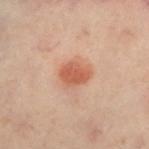Clinical summary:
About 3.5 mm across. From the right leg. A female subject, about 60 years old. Captured under cross-polarized illumination. Automated tile analysis of the lesion measured a mean CIELAB color near L≈50 a*≈24 b*≈30 and a normalized lesion–skin contrast near 8. It also reported an automated nevus-likeness rating near 100 out of 100. A close-up tile cropped from a whole-body skin photograph, about 15 mm across.